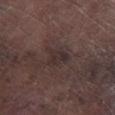workup = no biopsy performed (imaged during a skin exam)
subject = male, aged 73–77
body site = the right lower leg
tile lighting = white-light illumination
image source = 15 mm crop, total-body photography
size = ≈3 mm